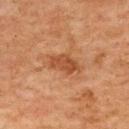No biopsy was performed on this lesion — it was imaged during a full skin examination and was not determined to be concerning.
A lesion tile, about 15 mm wide, cut from a 3D total-body photograph.
A female patient, approximately 60 years of age.
Captured under cross-polarized illumination.
The lesion is on the back.
Approximately 4 mm at its widest.